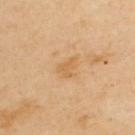{
  "automated_metrics": {
    "eccentricity": 0.7
  },
  "lesion_size": {
    "long_diameter_mm_approx": 2.5
  },
  "patient": {
    "sex": "male",
    "age_approx": 55
  },
  "site": "upper back",
  "image": {
    "source": "total-body photography crop",
    "field_of_view_mm": 15
  }
}